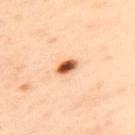Impression: No biopsy was performed on this lesion — it was imaged during a full skin examination and was not determined to be concerning. Context: About 2.5 mm across. The lesion is on the upper back. Imaged with cross-polarized lighting. A female subject, aged 38–42. A roughly 15 mm field-of-view crop from a total-body skin photograph.